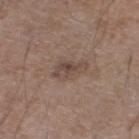Impression:
Recorded during total-body skin imaging; not selected for excision or biopsy.
Background:
The lesion's longest dimension is about 4 mm. This image is a 15 mm lesion crop taken from a total-body photograph. Captured under white-light illumination. The lesion is on the right lower leg. A male subject aged 58 to 62.